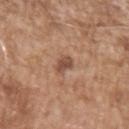{"biopsy_status": "not biopsied; imaged during a skin examination", "site": "left upper arm", "lesion_size": {"long_diameter_mm_approx": 3.0}, "automated_metrics": {"area_mm2_approx": 3.5, "eccentricity": 0.85, "shape_asymmetry": 0.35, "border_irregularity_0_10": 3.5, "color_variation_0_10": 1.5, "peripheral_color_asymmetry": 0.5}, "image": {"source": "total-body photography crop", "field_of_view_mm": 15}, "lighting": "white-light", "patient": {"sex": "male", "age_approx": 75}}